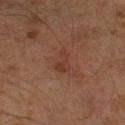Findings:
• biopsy status: total-body-photography surveillance lesion; no biopsy
• patient: male, aged around 60
• acquisition: ~15 mm crop, total-body skin-cancer survey
• anatomic site: the leg